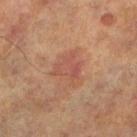Q: Was a biopsy performed?
A: imaged on a skin check; not biopsied
Q: What is the lesion's diameter?
A: about 3.5 mm
Q: What is the anatomic site?
A: the left lower leg
Q: Automated lesion metrics?
A: an automated nevus-likeness rating near 0 out of 100 and a detector confidence of about 100 out of 100 that the crop contains a lesion
Q: Illumination type?
A: cross-polarized illumination
Q: How was this image acquired?
A: 15 mm crop, total-body photography
Q: What are the patient's age and sex?
A: male, about 70 years old